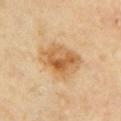Recorded during total-body skin imaging; not selected for excision or biopsy.
The lesion-visualizer software estimated a footprint of about 16 mm², an eccentricity of roughly 0.65, and a shape-asymmetry score of about 0.2 (0 = symmetric). It also reported a mean CIELAB color near L≈61 a*≈20 b*≈41, about 11 CIELAB-L* units darker than the surrounding skin, and a normalized border contrast of about 8. The analysis additionally found a nevus-likeness score of about 70/100.
This image is a 15 mm lesion crop taken from a total-body photograph.
From the left upper arm.
A female patient aged 58 to 62.
The recorded lesion diameter is about 5 mm.